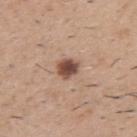notes=imaged on a skin check; not biopsied
automated metrics=about 16 CIELAB-L* units darker than the surrounding skin; a classifier nevus-likeness of about 90/100 and a detector confidence of about 100 out of 100 that the crop contains a lesion
patient=male, aged approximately 50
image source=~15 mm tile from a whole-body skin photo
illumination=white-light illumination
body site=the right upper arm
lesion size=about 2.5 mm